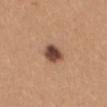notes = imaged on a skin check; not biopsied
size = about 3 mm
illumination = white-light
subject = female, aged approximately 40
image = 15 mm crop, total-body photography
location = the back